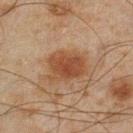Clinical impression:
Captured during whole-body skin photography for melanoma surveillance; the lesion was not biopsied.
Acquisition and patient details:
Located on the left lower leg. A 15 mm close-up tile from a total-body photography series done for melanoma screening. The subject is a male approximately 45 years of age. This is a cross-polarized tile.